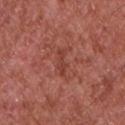| feature | finding |
|---|---|
| workup | imaged on a skin check; not biopsied |
| TBP lesion metrics | a footprint of about 2.5 mm²; an average lesion color of about L≈41 a*≈29 b*≈28 (CIELAB), roughly 7 lightness units darker than nearby skin, and a normalized border contrast of about 6; a classifier nevus-likeness of about 0/100 and lesion-presence confidence of about 100/100 |
| location | the upper back |
| lighting | white-light |
| size | ≈3 mm |
| subject | male, in their mid- to late 60s |
| image source | ~15 mm crop, total-body skin-cancer survey |